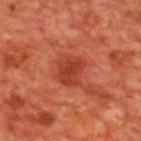Impression:
No biopsy was performed on this lesion — it was imaged during a full skin examination and was not determined to be concerning.
Background:
Longest diameter approximately 3.5 mm. A male patient approximately 70 years of age. From the upper back. This image is a 15 mm lesion crop taken from a total-body photograph. The tile uses cross-polarized illumination. The total-body-photography lesion software estimated a mean CIELAB color near L≈41 a*≈35 b*≈35, about 9 CIELAB-L* units darker than the surrounding skin, and a normalized lesion–skin contrast near 7. The analysis additionally found a border-irregularity rating of about 2/10, a within-lesion color-variation index near 2.5/10, and peripheral color asymmetry of about 0.5. And it measured a nevus-likeness score of about 20/100 and a detector confidence of about 100 out of 100 that the crop contains a lesion.